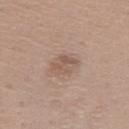Q: Was a biopsy performed?
A: imaged on a skin check; not biopsied
Q: What are the patient's age and sex?
A: female, roughly 60 years of age
Q: What lighting was used for the tile?
A: white-light illumination
Q: What kind of image is this?
A: ~15 mm tile from a whole-body skin photo
Q: What is the anatomic site?
A: the front of the torso